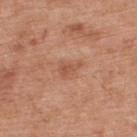Q: Is there a histopathology result?
A: total-body-photography surveillance lesion; no biopsy
Q: What are the patient's age and sex?
A: male, about 70 years old
Q: How large is the lesion?
A: ≈2.5 mm
Q: Lesion location?
A: the back
Q: What kind of image is this?
A: 15 mm crop, total-body photography
Q: Illumination type?
A: white-light
Q: What did automated image analysis measure?
A: a lesion area of about 2.5 mm², a shape eccentricity near 0.9, and a symmetry-axis asymmetry near 0.45; a mean CIELAB color near L≈53 a*≈24 b*≈33, about 8 CIELAB-L* units darker than the surrounding skin, and a lesion-to-skin contrast of about 6 (normalized; higher = more distinct); an automated nevus-likeness rating near 5 out of 100 and a lesion-detection confidence of about 100/100